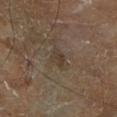{"biopsy_status": "not biopsied; imaged during a skin examination", "patient": {"sex": "male", "age_approx": 70}, "lighting": "cross-polarized", "lesion_size": {"long_diameter_mm_approx": 2.5}, "site": "right lower leg", "automated_metrics": {"area_mm2_approx": 3.5, "eccentricity": 0.75, "shape_asymmetry": 0.25, "border_irregularity_0_10": 2.5, "color_variation_0_10": 2.0, "peripheral_color_asymmetry": 0.5, "nevus_likeness_0_100": 0, "lesion_detection_confidence_0_100": 75}, "image": {"source": "total-body photography crop", "field_of_view_mm": 15}}